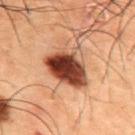Impression:
Captured during whole-body skin photography for melanoma surveillance; the lesion was not biopsied.
Clinical summary:
A region of skin cropped from a whole-body photographic capture, roughly 15 mm wide. The subject is a male aged 48 to 52. Located on the upper back. Measured at roughly 6 mm in maximum diameter.